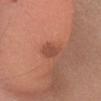Q: Was a biopsy performed?
A: catalogued during a skin exam; not biopsied
Q: Patient demographics?
A: female, aged around 35
Q: Lesion location?
A: the head or neck
Q: Illumination type?
A: white-light
Q: How was this image acquired?
A: 15 mm crop, total-body photography